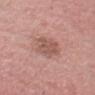A region of skin cropped from a whole-body photographic capture, roughly 15 mm wide. Automated tile analysis of the lesion measured a mean CIELAB color near L≈54 a*≈22 b*≈24, about 9 CIELAB-L* units darker than the surrounding skin, and a normalized lesion–skin contrast near 6.5. The analysis additionally found border irregularity of about 2 on a 0–10 scale and a peripheral color-asymmetry measure near 1. This is a white-light tile. The lesion is on the head or neck. A male subject, aged approximately 50. The lesion's longest dimension is about 3.5 mm.